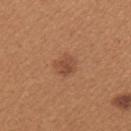Imaged during a routine full-body skin examination; the lesion was not biopsied and no histopathology is available.
The tile uses white-light illumination.
About 2.5 mm across.
Cropped from a whole-body photographic skin survey; the tile spans about 15 mm.
A female patient, approximately 40 years of age.
Automated tile analysis of the lesion measured a border-irregularity rating of about 2/10 and a color-variation rating of about 2/10. And it measured a classifier nevus-likeness of about 50/100 and a lesion-detection confidence of about 100/100.
From the arm.